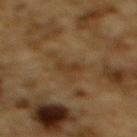workup=imaged on a skin check; not biopsied
automated metrics=a border-irregularity index near 4.5/10 and peripheral color asymmetry of about 0.5; a nevus-likeness score of about 0/100 and a detector confidence of about 60 out of 100 that the crop contains a lesion
subject=male, aged around 85 or older
diameter=about 3 mm
location=the upper back
imaging modality=total-body-photography crop, ~15 mm field of view
lighting=cross-polarized illumination